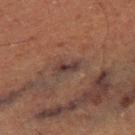Imaged during a routine full-body skin examination; the lesion was not biopsied and no histopathology is available.
Cropped from a whole-body photographic skin survey; the tile spans about 15 mm.
An algorithmic analysis of the crop reported a mean CIELAB color near L≈28 a*≈14 b*≈16, a lesion–skin lightness drop of about 8, and a normalized border contrast of about 10. The software also gave a lesion-detection confidence of about 75/100.
A male patient, aged 73–77.
This is a cross-polarized tile.
The lesion's longest dimension is about 2.5 mm.
On the right thigh.